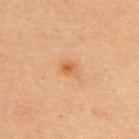This lesion was catalogued during total-body skin photography and was not selected for biopsy. The lesion is on the upper back. Cropped from a whole-body photographic skin survey; the tile spans about 15 mm. An algorithmic analysis of the crop reported a mean CIELAB color near L≈53 a*≈21 b*≈37, roughly 7 lightness units darker than nearby skin, and a normalized lesion–skin contrast near 6.5. The analysis additionally found a border-irregularity index near 3/10, a color-variation rating of about 3.5/10, and radial color variation of about 1. The subject is a female in their mid- to late 60s. Captured under cross-polarized illumination. Measured at roughly 2.5 mm in maximum diameter.